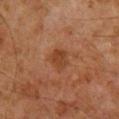Recorded during total-body skin imaging; not selected for excision or biopsy. Imaged with cross-polarized lighting. The patient is a male about 60 years old. On the back. A 15 mm crop from a total-body photograph taken for skin-cancer surveillance. The recorded lesion diameter is about 3 mm. An algorithmic analysis of the crop reported an outline eccentricity of about 0.6 (0 = round, 1 = elongated) and a shape-asymmetry score of about 0.25 (0 = symmetric). And it measured a border-irregularity index near 2.5/10, a within-lesion color-variation index near 1.5/10, and a peripheral color-asymmetry measure near 0.5. It also reported a lesion-detection confidence of about 100/100.